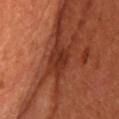This lesion was catalogued during total-body skin photography and was not selected for biopsy. Cropped from a whole-body photographic skin survey; the tile spans about 15 mm. The lesion is located on the head or neck. Imaged with cross-polarized lighting. The subject is a female approximately 65 years of age. An algorithmic analysis of the crop reported an average lesion color of about L≈33 a*≈28 b*≈31 (CIELAB), roughly 9 lightness units darker than nearby skin, and a normalized lesion–skin contrast near 8. The analysis additionally found a peripheral color-asymmetry measure near 1. The software also gave a nevus-likeness score of about 0/100 and a lesion-detection confidence of about 85/100.